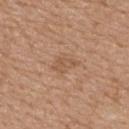Assessment: Imaged during a routine full-body skin examination; the lesion was not biopsied and no histopathology is available. Acquisition and patient details: An algorithmic analysis of the crop reported a classifier nevus-likeness of about 0/100 and lesion-presence confidence of about 100/100. Cropped from a total-body skin-imaging series; the visible field is about 15 mm. A female subject, aged 68 to 72. About 3 mm across. Captured under white-light illumination. Located on the upper back.